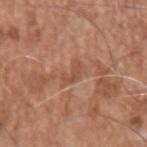The lesion was photographed on a routine skin check and not biopsied; there is no pathology result. A male patient, in their mid-70s. Measured at roughly 3 mm in maximum diameter. The lesion is located on the left upper arm. Captured under white-light illumination. Cropped from a total-body skin-imaging series; the visible field is about 15 mm.